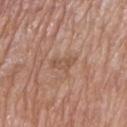notes: total-body-photography surveillance lesion; no biopsy | site: the left upper arm | imaging modality: ~15 mm crop, total-body skin-cancer survey | diameter: ≈3 mm | subject: male, aged 68 to 72.